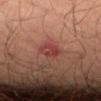Impression:
Captured during whole-body skin photography for melanoma surveillance; the lesion was not biopsied.
Context:
Imaged with cross-polarized lighting. This image is a 15 mm lesion crop taken from a total-body photograph. From the lower back. The lesion's longest dimension is about 2.5 mm. Automated image analysis of the tile measured an average lesion color of about L≈37 a*≈26 b*≈24 (CIELAB), roughly 7 lightness units darker than nearby skin, and a normalized border contrast of about 6.5. The software also gave border irregularity of about 2 on a 0–10 scale, a color-variation rating of about 5.5/10, and radial color variation of about 2. It also reported a classifier nevus-likeness of about 10/100 and a lesion-detection confidence of about 100/100. The subject is a male in their mid- to late 30s.